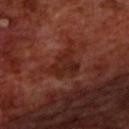• notes — imaged on a skin check; not biopsied
• TBP lesion metrics — an area of roughly 11 mm², an outline eccentricity of about 0.65 (0 = round, 1 = elongated), and a shape-asymmetry score of about 0.55 (0 = symmetric); a lesion color around L≈25 a*≈23 b*≈25 in CIELAB
• site — the upper back
• patient — male, approximately 70 years of age
• image source — ~15 mm crop, total-body skin-cancer survey
• size — ≈5.5 mm
• illumination — cross-polarized illumination